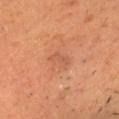Impression: This lesion was catalogued during total-body skin photography and was not selected for biopsy. Background: The patient is a male aged 48 to 52. From the head or neck. This is a cross-polarized tile. Cropped from a total-body skin-imaging series; the visible field is about 15 mm. About 4 mm across.